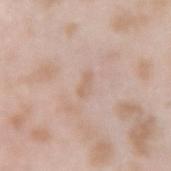Assessment: The lesion was photographed on a routine skin check and not biopsied; there is no pathology result. Image and clinical context: The lesion is on the right forearm. This is a white-light tile. The subject is a female aged 23–27. Cropped from a whole-body photographic skin survey; the tile spans about 15 mm.